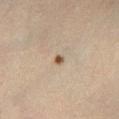Assessment:
No biopsy was performed on this lesion — it was imaged during a full skin examination and was not determined to be concerning.
Clinical summary:
Longest diameter approximately 1.5 mm. A male patient, aged around 30. On the left lower leg. The tile uses cross-polarized illumination. This image is a 15 mm lesion crop taken from a total-body photograph.